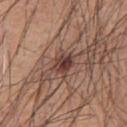Assessment: Imaged during a routine full-body skin examination; the lesion was not biopsied and no histopathology is available. Acquisition and patient details: The total-body-photography lesion software estimated a lesion area of about 6 mm² and a shape eccentricity near 0.65. It also reported border irregularity of about 3.5 on a 0–10 scale, internal color variation of about 5 on a 0–10 scale, and radial color variation of about 1.5. This image is a 15 mm lesion crop taken from a total-body photograph. Captured under white-light illumination. A male patient, aged approximately 60. Approximately 3 mm at its widest. From the chest.